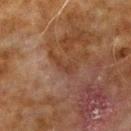Clinical impression:
Recorded during total-body skin imaging; not selected for excision or biopsy.
Acquisition and patient details:
The subject is a male aged approximately 70. On the right upper arm. A region of skin cropped from a whole-body photographic capture, roughly 15 mm wide.